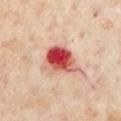{
  "biopsy_status": "not biopsied; imaged during a skin examination",
  "patient": {
    "sex": "female",
    "age_approx": 60
  },
  "image": {
    "source": "total-body photography crop",
    "field_of_view_mm": 15
  },
  "site": "front of the torso"
}